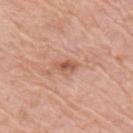The lesion was photographed on a routine skin check and not biopsied; there is no pathology result. Measured at roughly 3 mm in maximum diameter. A male subject, aged around 80. The lesion is on the right upper arm. The total-body-photography lesion software estimated an area of roughly 3.5 mm² and an outline eccentricity of about 0.85 (0 = round, 1 = elongated). The software also gave an average lesion color of about L≈56 a*≈25 b*≈32 (CIELAB), a lesion–skin lightness drop of about 10, and a normalized border contrast of about 7. The analysis additionally found a border-irregularity rating of about 3.5/10 and internal color variation of about 3 on a 0–10 scale. It also reported a classifier nevus-likeness of about 5/100 and a lesion-detection confidence of about 100/100. A 15 mm crop from a total-body photograph taken for skin-cancer surveillance.